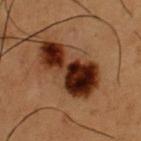  biopsy_status: not biopsied; imaged during a skin examination
  patient:
    sex: male
    age_approx: 50
  lighting: cross-polarized
  lesion_size:
    long_diameter_mm_approx: 7.5
  automated_metrics:
    area_mm2_approx: 20.0
    eccentricity: 0.9
    border_irregularity_0_10: 5.5
    color_variation_0_10: 8.0
    peripheral_color_asymmetry: 2.5
  site: chest
  image:
    source: total-body photography crop
    field_of_view_mm: 15